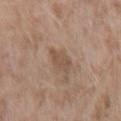biopsy_status: not biopsied; imaged during a skin examination
lesion_size:
  long_diameter_mm_approx: 3.0
patient:
  sex: female
  age_approx: 75
site: left forearm
image:
  source: total-body photography crop
  field_of_view_mm: 15
lighting: white-light